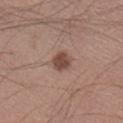{
  "biopsy_status": "not biopsied; imaged during a skin examination",
  "site": "right lower leg",
  "image": {
    "source": "total-body photography crop",
    "field_of_view_mm": 15
  },
  "lesion_size": {
    "long_diameter_mm_approx": 2.5
  },
  "automated_metrics": {
    "area_mm2_approx": 5.0,
    "shape_asymmetry": 0.2,
    "cielab_L": 46,
    "cielab_a": 20,
    "cielab_b": 24,
    "vs_skin_darker_L": 12.0,
    "vs_skin_contrast_norm": 9.0,
    "border_irregularity_0_10": 1.5,
    "nevus_likeness_0_100": 95,
    "lesion_detection_confidence_0_100": 100
  },
  "lighting": "white-light",
  "patient": {
    "sex": "male",
    "age_approx": 40
  }
}